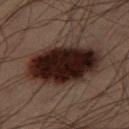Impression:
No biopsy was performed on this lesion — it was imaged during a full skin examination and was not determined to be concerning.
Background:
Measured at roughly 8.5 mm in maximum diameter. Captured under cross-polarized illumination. A male patient, aged 53–57. Located on the leg. Cropped from a whole-body photographic skin survey; the tile spans about 15 mm.